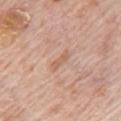  biopsy_status: not biopsied; imaged during a skin examination
  patient:
    sex: male
    age_approx: 80
  site: chest
  lighting: white-light
  image:
    source: total-body photography crop
    field_of_view_mm: 15